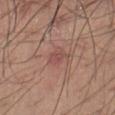Part of a total-body skin-imaging series; this lesion was reviewed on a skin check and was not flagged for biopsy. A male patient, in their mid-50s. A 15 mm crop from a total-body photograph taken for skin-cancer surveillance. On the right thigh. About 3 mm across. Captured under white-light illumination.